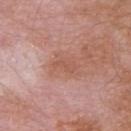<case>
<biopsy_status>not biopsied; imaged during a skin examination</biopsy_status>
<automated_metrics>
  <cielab_L>55</cielab_L>
  <cielab_a>23</cielab_a>
  <cielab_b>29</cielab_b>
  <vs_skin_darker_L>6.0</vs_skin_darker_L>
  <vs_skin_contrast_norm>5.0</vs_skin_contrast_norm>
  <border_irregularity_0_10>5.0</border_irregularity_0_10>
  <color_variation_0_10>0.5</color_variation_0_10>
  <nevus_likeness_0_100>0</nevus_likeness_0_100>
  <lesion_detection_confidence_0_100>100</lesion_detection_confidence_0_100>
</automated_metrics>
<lighting>white-light</lighting>
<site>right upper arm</site>
<patient>
  <sex>male</sex>
  <age_approx>80</age_approx>
</patient>
<lesion_size>
  <long_diameter_mm_approx>3.0</long_diameter_mm_approx>
</lesion_size>
<image>
  <source>total-body photography crop</source>
  <field_of_view_mm>15</field_of_view_mm>
</image>
</case>